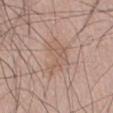Part of a total-body skin-imaging series; this lesion was reviewed on a skin check and was not flagged for biopsy. A male patient, in their mid-70s. A lesion tile, about 15 mm wide, cut from a 3D total-body photograph. The total-body-photography lesion software estimated a lesion color around L≈58 a*≈17 b*≈27 in CIELAB and a lesion–skin lightness drop of about 6. The analysis additionally found a border-irregularity rating of about 5.5/10, internal color variation of about 2.5 on a 0–10 scale, and peripheral color asymmetry of about 1. Longest diameter approximately 5 mm. On the abdomen.